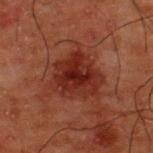Image and clinical context:
Captured under cross-polarized illumination. A region of skin cropped from a whole-body photographic capture, roughly 15 mm wide. The lesion is located on the upper back. The lesion-visualizer software estimated a lesion area of about 18 mm², an eccentricity of roughly 0.55, and two-axis asymmetry of about 0.25. The analysis additionally found a lesion color around L≈21 a*≈23 b*≈23 in CIELAB, roughly 8 lightness units darker than nearby skin, and a normalized border contrast of about 9. It also reported border irregularity of about 3 on a 0–10 scale, a color-variation rating of about 4.5/10, and radial color variation of about 1. It also reported a classifier nevus-likeness of about 75/100 and a lesion-detection confidence of about 100/100. Measured at roughly 5.5 mm in maximum diameter. A male subject, in their 50s.